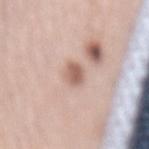Q: Was a biopsy performed?
A: imaged on a skin check; not biopsied
Q: How was this image acquired?
A: ~15 mm tile from a whole-body skin photo
Q: What is the lesion's diameter?
A: ~3 mm (longest diameter)
Q: Lesion location?
A: the mid back
Q: What are the patient's age and sex?
A: male, approximately 65 years of age